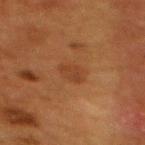patient: female, aged 48–52
tile lighting: cross-polarized
TBP lesion metrics: an area of roughly 5 mm² and a shape-asymmetry score of about 0.15 (0 = symmetric); lesion-presence confidence of about 100/100
image: 15 mm crop, total-body photography
body site: the mid back
diameter: ~3 mm (longest diameter)